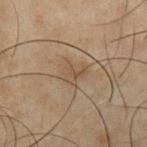Imaged during a routine full-body skin examination; the lesion was not biopsied and no histopathology is available.
This is a cross-polarized tile.
Approximately 3 mm at its widest.
On the front of the torso.
A close-up tile cropped from a whole-body skin photograph, about 15 mm across.
A male patient roughly 50 years of age.